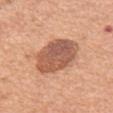Assessment: Part of a total-body skin-imaging series; this lesion was reviewed on a skin check and was not flagged for biopsy. Clinical summary: Measured at roughly 6 mm in maximum diameter. This image is a 15 mm lesion crop taken from a total-body photograph. A male patient, aged 63 to 67. The lesion is located on the abdomen.